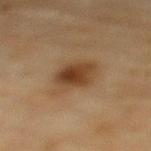Q: Was a biopsy performed?
A: catalogued during a skin exam; not biopsied
Q: What kind of image is this?
A: ~15 mm tile from a whole-body skin photo
Q: What did automated image analysis measure?
A: internal color variation of about 5.5 on a 0–10 scale and a peripheral color-asymmetry measure near 1.5; an automated nevus-likeness rating near 95 out of 100 and lesion-presence confidence of about 100/100
Q: Where on the body is the lesion?
A: the upper back
Q: What are the patient's age and sex?
A: female, about 80 years old
Q: What lighting was used for the tile?
A: cross-polarized